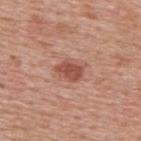| field | value |
|---|---|
| follow-up | total-body-photography surveillance lesion; no biopsy |
| patient | male, about 75 years old |
| location | the upper back |
| image | ~15 mm tile from a whole-body skin photo |
| size | about 3.5 mm |
| lighting | white-light illumination |